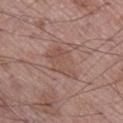Assessment: No biopsy was performed on this lesion — it was imaged during a full skin examination and was not determined to be concerning. Acquisition and patient details: A 15 mm close-up extracted from a 3D total-body photography capture. A male subject roughly 70 years of age. The lesion is located on the right lower leg.